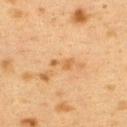The lesion was photographed on a routine skin check and not biopsied; there is no pathology result. On the upper back. A 15 mm close-up extracted from a 3D total-body photography capture. The subject is a female approximately 40 years of age.